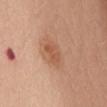Q: Was this lesion biopsied?
A: total-body-photography surveillance lesion; no biopsy
Q: How was this image acquired?
A: ~15 mm crop, total-body skin-cancer survey
Q: Patient demographics?
A: female, approximately 50 years of age
Q: Where on the body is the lesion?
A: the chest